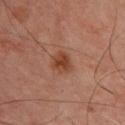Assessment: Captured during whole-body skin photography for melanoma surveillance; the lesion was not biopsied. Context: Approximately 3 mm at its widest. A 15 mm crop from a total-body photograph taken for skin-cancer surveillance. On the chest. Captured under cross-polarized illumination. A male patient, aged approximately 50.